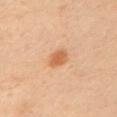Q: Was a biopsy performed?
A: catalogued during a skin exam; not biopsied
Q: Who is the patient?
A: female, aged around 40
Q: How was this image acquired?
A: ~15 mm tile from a whole-body skin photo
Q: Where on the body is the lesion?
A: the left upper arm